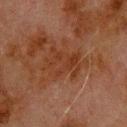Q: Illumination type?
A: cross-polarized illumination
Q: Automated lesion metrics?
A: a footprint of about 14 mm² and an eccentricity of roughly 0.8; an average lesion color of about L≈29 a*≈19 b*≈26 (CIELAB), a lesion–skin lightness drop of about 5, and a normalized border contrast of about 6; border irregularity of about 6 on a 0–10 scale, a color-variation rating of about 3/10, and radial color variation of about 1; an automated nevus-likeness rating near 0 out of 100 and lesion-presence confidence of about 100/100
Q: Patient demographics?
A: male, aged 78–82
Q: Lesion size?
A: ≈5.5 mm
Q: What kind of image is this?
A: ~15 mm crop, total-body skin-cancer survey
Q: What is the anatomic site?
A: the back